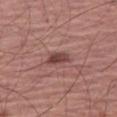site: right thigh
lighting: white-light
patient:
  sex: male
  age_approx: 65
image:
  source: total-body photography crop
  field_of_view_mm: 15
automated_metrics:
  cielab_L: 43
  cielab_a: 23
  cielab_b: 22
  vs_skin_darker_L: 12.0
  vs_skin_contrast_norm: 9.0
  border_irregularity_0_10: 2.0
  color_variation_0_10: 3.5
  peripheral_color_asymmetry: 1.5